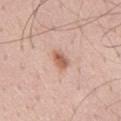  biopsy_status: not biopsied; imaged during a skin examination
  site: chest
  lesion_size:
    long_diameter_mm_approx: 3.0
  image:
    source: total-body photography crop
    field_of_view_mm: 15
  lighting: white-light
  patient:
    sex: male
    age_approx: 50